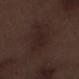follow-up: total-body-photography surveillance lesion; no biopsy
image-analysis metrics: a footprint of about 8.5 mm², an outline eccentricity of about 0.85 (0 = round, 1 = elongated), and a shape-asymmetry score of about 0.35 (0 = symmetric); a lesion color around L≈21 a*≈14 b*≈16 in CIELAB, about 4 CIELAB-L* units darker than the surrounding skin, and a normalized border contrast of about 6; a border-irregularity rating of about 3.5/10, internal color variation of about 1.5 on a 0–10 scale, and a peripheral color-asymmetry measure near 0.5; a nevus-likeness score of about 0/100 and a lesion-detection confidence of about 100/100
anatomic site: the leg
size: about 5 mm
image source: ~15 mm tile from a whole-body skin photo
tile lighting: white-light
patient: male, in their 70s